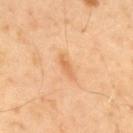Assessment:
No biopsy was performed on this lesion — it was imaged during a full skin examination and was not determined to be concerning.
Clinical summary:
A male subject, aged approximately 40. Cropped from a whole-body photographic skin survey; the tile spans about 15 mm. The lesion-visualizer software estimated an area of roughly 3 mm², an outline eccentricity of about 0.9 (0 = round, 1 = elongated), and two-axis asymmetry of about 0.3. And it measured roughly 8 lightness units darker than nearby skin and a normalized lesion–skin contrast near 6. The analysis additionally found a border-irregularity rating of about 3/10, internal color variation of about 1 on a 0–10 scale, and a peripheral color-asymmetry measure near 0.5. The analysis additionally found a nevus-likeness score of about 0/100 and a lesion-detection confidence of about 100/100. The recorded lesion diameter is about 3 mm. The lesion is on the mid back.